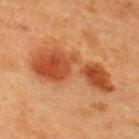This lesion was catalogued during total-body skin photography and was not selected for biopsy. Captured under cross-polarized illumination. On the upper back. The total-body-photography lesion software estimated a footprint of about 26 mm², an outline eccentricity of about 0.95 (0 = round, 1 = elongated), and a shape-asymmetry score of about 0.45 (0 = symmetric). It also reported a classifier nevus-likeness of about 100/100 and a lesion-detection confidence of about 100/100. A 15 mm crop from a total-body photograph taken for skin-cancer surveillance. The recorded lesion diameter is about 10 mm. A female subject, aged 38 to 42.